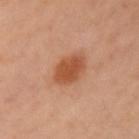No biopsy was performed on this lesion — it was imaged during a full skin examination and was not determined to be concerning.